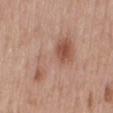Assessment:
Imaged during a routine full-body skin examination; the lesion was not biopsied and no histopathology is available.
Acquisition and patient details:
A 15 mm crop from a total-body photograph taken for skin-cancer surveillance. Approximately 9 mm at its widest. Captured under white-light illumination. A male subject about 70 years old. On the mid back.